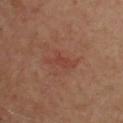biopsy status — imaged on a skin check; not biopsied | lighting — cross-polarized illumination | TBP lesion metrics — a footprint of about 4 mm², an outline eccentricity of about 0.9 (0 = round, 1 = elongated), and two-axis asymmetry of about 0.4 | imaging modality — total-body-photography crop, ~15 mm field of view | size — ≈3.5 mm | location — the chest | patient — male, aged around 50.